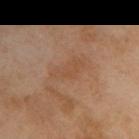Captured during whole-body skin photography for melanoma surveillance; the lesion was not biopsied. From the right upper arm. A male patient about 70 years old. A 15 mm crop from a total-body photograph taken for skin-cancer surveillance.